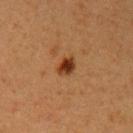Clinical impression:
This lesion was catalogued during total-body skin photography and was not selected for biopsy.
Context:
The subject is a female roughly 40 years of age. A lesion tile, about 15 mm wide, cut from a 3D total-body photograph. On the left upper arm. The total-body-photography lesion software estimated a border-irregularity index near 2/10, a within-lesion color-variation index near 3.5/10, and a peripheral color-asymmetry measure near 1. The analysis additionally found a classifier nevus-likeness of about 100/100 and a lesion-detection confidence of about 100/100. Imaged with cross-polarized lighting. The lesion's longest dimension is about 2.5 mm.